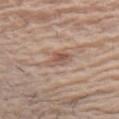The lesion was tiled from a total-body skin photograph and was not biopsied. The lesion is on the chest. Longest diameter approximately 3 mm. A close-up tile cropped from a whole-body skin photograph, about 15 mm across. The subject is a female in their mid- to late 60s. This is a white-light tile. Automated image analysis of the tile measured a lesion area of about 4.5 mm², an eccentricity of roughly 0.7, and two-axis asymmetry of about 0.3. The software also gave a color-variation rating of about 4/10 and a peripheral color-asymmetry measure near 1.